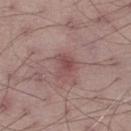Image and clinical context: A male subject about 70 years old. From the left thigh. A lesion tile, about 15 mm wide, cut from a 3D total-body photograph. Captured under white-light illumination. Approximately 2.5 mm at its widest.